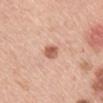The lesion was tiled from a total-body skin photograph and was not biopsied. A female subject in their mid-60s. Cropped from a total-body skin-imaging series; the visible field is about 15 mm. Located on the mid back.